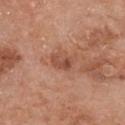Notes:
- notes · no biopsy performed (imaged during a skin exam)
- anatomic site · the chest
- subject · female, in their mid-60s
- automated metrics · a footprint of about 4 mm²; a lesion–skin lightness drop of about 10; an automated nevus-likeness rating near 0 out of 100 and a lesion-detection confidence of about 100/100
- image source · ~15 mm crop, total-body skin-cancer survey